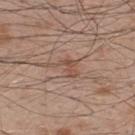- follow-up: total-body-photography surveillance lesion; no biopsy
- automated metrics: a shape eccentricity near 0.85 and a shape-asymmetry score of about 0.65 (0 = symmetric); border irregularity of about 8 on a 0–10 scale and peripheral color asymmetry of about 0.5; an automated nevus-likeness rating near 0 out of 100 and a detector confidence of about 100 out of 100 that the crop contains a lesion
- acquisition: total-body-photography crop, ~15 mm field of view
- patient: male, aged approximately 50
- anatomic site: the upper back